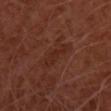Q: Was this lesion biopsied?
A: no biopsy performed (imaged during a skin exam)
Q: What kind of image is this?
A: 15 mm crop, total-body photography
Q: Lesion size?
A: ≈4 mm
Q: Who is the patient?
A: male, aged approximately 50
Q: Illumination type?
A: cross-polarized
Q: What is the anatomic site?
A: the chest